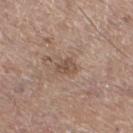Part of a total-body skin-imaging series; this lesion was reviewed on a skin check and was not flagged for biopsy.
The lesion is located on the right thigh.
Longest diameter approximately 2.5 mm.
The lesion-visualizer software estimated an area of roughly 4 mm² and an eccentricity of roughly 0.7. The analysis additionally found a mean CIELAB color near L≈50 a*≈17 b*≈26 and a lesion-to-skin contrast of about 6 (normalized; higher = more distinct). It also reported a border-irregularity index near 3/10.
A region of skin cropped from a whole-body photographic capture, roughly 15 mm wide.
Captured under white-light illumination.
The subject is a male aged 63 to 67.